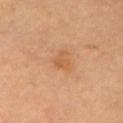Assessment: Imaged during a routine full-body skin examination; the lesion was not biopsied and no histopathology is available. Context: The patient is a female in their mid-60s. This is a cross-polarized tile. A lesion tile, about 15 mm wide, cut from a 3D total-body photograph. The lesion is on the arm.